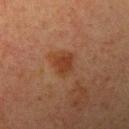Q: Was a biopsy performed?
A: catalogued during a skin exam; not biopsied
Q: What lighting was used for the tile?
A: cross-polarized
Q: Who is the patient?
A: female, aged around 55
Q: Where on the body is the lesion?
A: the left upper arm
Q: Lesion size?
A: ~3 mm (longest diameter)
Q: How was this image acquired?
A: ~15 mm crop, total-body skin-cancer survey
Q: What did automated image analysis measure?
A: a lesion area of about 5.5 mm², an outline eccentricity of about 0.55 (0 = round, 1 = elongated), and a symmetry-axis asymmetry near 0.3